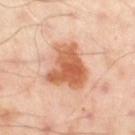Imaged during a routine full-body skin examination; the lesion was not biopsied and no histopathology is available. A male patient aged 48–52. The lesion is on the left thigh. The tile uses cross-polarized illumination. Cropped from a whole-body photographic skin survey; the tile spans about 15 mm. The recorded lesion diameter is about 5.5 mm.